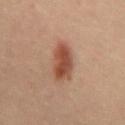Notes:
* biopsy status — catalogued during a skin exam; not biopsied
* body site — the chest
* imaging modality — ~15 mm tile from a whole-body skin photo
* lighting — cross-polarized illumination
* subject — male, in their 40s
* diameter — ≈4.5 mm